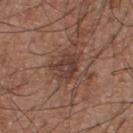Imaged during a routine full-body skin examination; the lesion was not biopsied and no histopathology is available.
The lesion's longest dimension is about 3.5 mm.
A lesion tile, about 15 mm wide, cut from a 3D total-body photograph.
Imaged with white-light lighting.
Located on the front of the torso.
A male patient roughly 55 years of age.